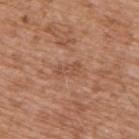The lesion was tiled from a total-body skin photograph and was not biopsied. Cropped from a total-body skin-imaging series; the visible field is about 15 mm. A male patient, in their mid-70s. The lesion is on the right upper arm. This is a white-light tile.